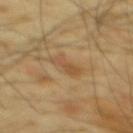Impression:
This lesion was catalogued during total-body skin photography and was not selected for biopsy.
Image and clinical context:
The total-body-photography lesion software estimated an average lesion color of about L≈52 a*≈18 b*≈36 (CIELAB). A male patient aged approximately 65. The lesion is on the back. The lesion's longest dimension is about 3.5 mm. The tile uses cross-polarized illumination. A 15 mm crop from a total-body photograph taken for skin-cancer surveillance.